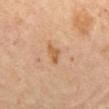Recorded during total-body skin imaging; not selected for excision or biopsy.
Imaged with cross-polarized lighting.
The lesion is located on the mid back.
A female subject aged around 65.
Automated image analysis of the tile measured a border-irregularity rating of about 3.5/10, a color-variation rating of about 1/10, and radial color variation of about 0.5. It also reported a classifier nevus-likeness of about 10/100 and a lesion-detection confidence of about 100/100.
Cropped from a whole-body photographic skin survey; the tile spans about 15 mm.
About 2.5 mm across.